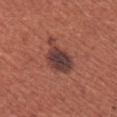| key | value |
|---|---|
| subject | female, about 35 years old |
| body site | the left upper arm |
| lighting | white-light illumination |
| diameter | ≈5.5 mm |
| acquisition | ~15 mm crop, total-body skin-cancer survey |